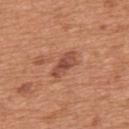The lesion was photographed on a routine skin check and not biopsied; there is no pathology result. A roughly 15 mm field-of-view crop from a total-body skin photograph. This is a white-light tile. From the upper back. The patient is a male in their mid- to late 60s. The lesion-visualizer software estimated an automated nevus-likeness rating near 10 out of 100 and lesion-presence confidence of about 100/100. The recorded lesion diameter is about 4 mm.